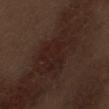patient: male, approximately 70 years of age
automated lesion analysis: an average lesion color of about L≈21 a*≈16 b*≈19 (CIELAB), a lesion–skin lightness drop of about 5, and a lesion-to-skin contrast of about 7 (normalized; higher = more distinct); a detector confidence of about 95 out of 100 that the crop contains a lesion
location: the abdomen
imaging modality: 15 mm crop, total-body photography
illumination: white-light